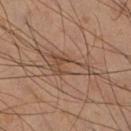{
  "biopsy_status": "not biopsied; imaged during a skin examination",
  "lighting": "cross-polarized",
  "patient": {
    "sex": "male",
    "age_approx": 50
  },
  "lesion_size": {
    "long_diameter_mm_approx": 6.0
  },
  "image": {
    "source": "total-body photography crop",
    "field_of_view_mm": 15
  },
  "site": "right lower leg"
}